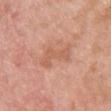site: chest
image:
  source: total-body photography crop
  field_of_view_mm: 15
patient:
  sex: female
  age_approx: 40